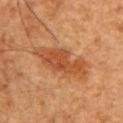Impression: Recorded during total-body skin imaging; not selected for excision or biopsy. Image and clinical context: Automated image analysis of the tile measured about 10 CIELAB-L* units darker than the surrounding skin and a normalized lesion–skin contrast near 7.5. Imaged with cross-polarized lighting. The patient is a male aged 43 to 47. A region of skin cropped from a whole-body photographic capture, roughly 15 mm wide. From the chest. Longest diameter approximately 7 mm.